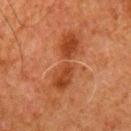notes: catalogued during a skin exam; not biopsied | anatomic site: the mid back | imaging modality: ~15 mm tile from a whole-body skin photo | subject: male, aged around 80.